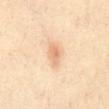Assessment:
Imaged during a routine full-body skin examination; the lesion was not biopsied and no histopathology is available.
Acquisition and patient details:
From the front of the torso. The patient is a female about 40 years old. A 15 mm close-up extracted from a 3D total-body photography capture. The total-body-photography lesion software estimated border irregularity of about 2 on a 0–10 scale, a within-lesion color-variation index near 2.5/10, and radial color variation of about 1. Imaged with cross-polarized lighting.